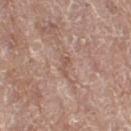This lesion was catalogued during total-body skin photography and was not selected for biopsy.
Cropped from a total-body skin-imaging series; the visible field is about 15 mm.
A female subject, about 75 years old.
Located on the leg.
The tile uses white-light illumination.